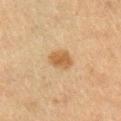Notes:
* biopsy status: total-body-photography surveillance lesion; no biopsy
* image-analysis metrics: a lesion–skin lightness drop of about 9 and a normalized border contrast of about 7.5; a border-irregularity rating of about 1.5/10 and a color-variation rating of about 2.5/10; a classifier nevus-likeness of about 95/100
* location: the left upper arm
* subject: male, aged 63 to 67
* lighting: cross-polarized illumination
* size: about 3 mm
* imaging modality: total-body-photography crop, ~15 mm field of view